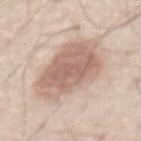Case summary:
– workup: no biopsy performed (imaged during a skin exam)
– size: ≈8 mm
– acquisition: ~15 mm crop, total-body skin-cancer survey
– anatomic site: the abdomen
– patient: male, approximately 70 years of age
– lighting: white-light illumination